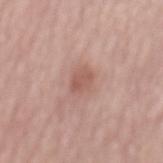notes — total-body-photography surveillance lesion; no biopsy
image source — total-body-photography crop, ~15 mm field of view
automated lesion analysis — a classifier nevus-likeness of about 10/100 and a detector confidence of about 100 out of 100 that the crop contains a lesion
subject — male, aged 73 to 77
size — ~3 mm (longest diameter)
tile lighting — white-light
body site — the mid back